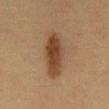This lesion was catalogued during total-body skin photography and was not selected for biopsy. On the mid back. The recorded lesion diameter is about 6.5 mm. A region of skin cropped from a whole-body photographic capture, roughly 15 mm wide. The tile uses cross-polarized illumination. A male subject, aged 33 to 37.